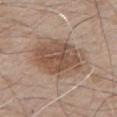Impression:
Captured during whole-body skin photography for melanoma surveillance; the lesion was not biopsied.
Context:
A lesion tile, about 15 mm wide, cut from a 3D total-body photograph. From the chest. The recorded lesion diameter is about 6.5 mm. A male subject approximately 65 years of age.